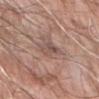Findings:
– workup — total-body-photography surveillance lesion; no biopsy
– image — total-body-photography crop, ~15 mm field of view
– TBP lesion metrics — an area of roughly 5.5 mm², an outline eccentricity of about 0.8 (0 = round, 1 = elongated), and a symmetry-axis asymmetry near 0.25; an average lesion color of about L≈51 a*≈17 b*≈22 (CIELAB), a lesion–skin lightness drop of about 8, and a lesion-to-skin contrast of about 6 (normalized; higher = more distinct); border irregularity of about 2.5 on a 0–10 scale and a peripheral color-asymmetry measure near 2
– subject — male, in their 60s
– body site — the right forearm
– lighting — white-light
– diameter — about 3 mm